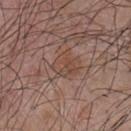No biopsy was performed on this lesion — it was imaged during a full skin examination and was not determined to be concerning. An algorithmic analysis of the crop reported a lesion color around L≈46 a*≈19 b*≈26 in CIELAB, a lesion–skin lightness drop of about 5, and a lesion-to-skin contrast of about 5.5 (normalized; higher = more distinct). The software also gave a classifier nevus-likeness of about 0/100 and lesion-presence confidence of about 100/100. The lesion is on the chest. A male subject aged around 55. Measured at roughly 3 mm in maximum diameter. This image is a 15 mm lesion crop taken from a total-body photograph.